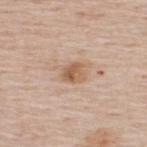biopsy status — imaged on a skin check; not biopsied | body site — the upper back | image source — total-body-photography crop, ~15 mm field of view | tile lighting — white-light illumination | subject — male, approximately 60 years of age | automated metrics — an area of roughly 4.5 mm² and a shape-asymmetry score of about 0.25 (0 = symmetric); a normalized border contrast of about 7.5; border irregularity of about 2 on a 0–10 scale, a color-variation rating of about 4.5/10, and peripheral color asymmetry of about 2; a detector confidence of about 100 out of 100 that the crop contains a lesion.